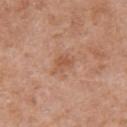This lesion was catalogued during total-body skin photography and was not selected for biopsy. A female patient roughly 75 years of age. Located on the back. This image is a 15 mm lesion crop taken from a total-body photograph. The lesion-visualizer software estimated an eccentricity of roughly 0.7 and two-axis asymmetry of about 0.45. And it measured an average lesion color of about L≈54 a*≈23 b*≈33 (CIELAB) and roughly 8 lightness units darker than nearby skin. It also reported lesion-presence confidence of about 100/100. Longest diameter approximately 2.5 mm.